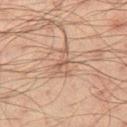The lesion was photographed on a routine skin check and not biopsied; there is no pathology result.
The total-body-photography lesion software estimated a lesion color around L≈53 a*≈18 b*≈27 in CIELAB, about 8 CIELAB-L* units darker than the surrounding skin, and a normalized lesion–skin contrast near 5.5. The software also gave a border-irregularity index near 7.5/10, internal color variation of about 3 on a 0–10 scale, and a peripheral color-asymmetry measure near 1.
A male subject aged 33 to 37.
A 15 mm close-up extracted from a 3D total-body photography capture.
Approximately 3.5 mm at its widest.
This is a cross-polarized tile.
The lesion is on the left thigh.